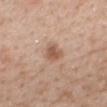follow-up: catalogued during a skin exam; not biopsied | image source: total-body-photography crop, ~15 mm field of view | location: the mid back | tile lighting: white-light illumination | subject: female, aged 48 to 52 | size: ≈2.5 mm.